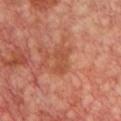Clinical impression:
Imaged during a routine full-body skin examination; the lesion was not biopsied and no histopathology is available.
Acquisition and patient details:
A female patient, approximately 45 years of age. A 15 mm close-up tile from a total-body photography series done for melanoma screening. Located on the chest. This is a cross-polarized tile.